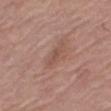biopsy_status: not biopsied; imaged during a skin examination
lesion_size:
  long_diameter_mm_approx: 2.5
site: right thigh
lighting: white-light
image:
  source: total-body photography crop
  field_of_view_mm: 15
automated_metrics:
  area_mm2_approx: 2.5
  eccentricity: 0.9
  shape_asymmetry: 0.35
  vs_skin_darker_L: 7.0
  vs_skin_contrast_norm: 5.0
  nevus_likeness_0_100: 0
patient:
  sex: female
  age_approx: 60